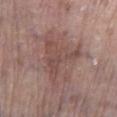No biopsy was performed on this lesion — it was imaged during a full skin examination and was not determined to be concerning. Captured under white-light illumination. A female patient aged approximately 85 or older. From the left lower leg. A 15 mm close-up tile from a total-body photography series done for melanoma screening. Measured at roughly 7.5 mm in maximum diameter.